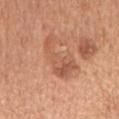Impression:
Imaged during a routine full-body skin examination; the lesion was not biopsied and no histopathology is available.
Context:
A lesion tile, about 15 mm wide, cut from a 3D total-body photograph. The tile uses white-light illumination. The subject is a male in their mid-60s. The recorded lesion diameter is about 6.5 mm. Located on the mid back.